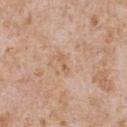Notes:
– workup: imaged on a skin check; not biopsied